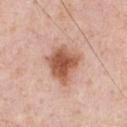The lesion was tiled from a total-body skin photograph and was not biopsied.
A male patient about 50 years old.
The lesion is on the front of the torso.
Imaged with white-light lighting.
Automated image analysis of the tile measured a lesion area of about 14 mm² and a shape-asymmetry score of about 0.3 (0 = symmetric). The software also gave border irregularity of about 3 on a 0–10 scale and radial color variation of about 1.
Measured at roughly 4.5 mm in maximum diameter.
A lesion tile, about 15 mm wide, cut from a 3D total-body photograph.